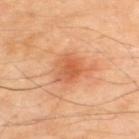Findings:
• follow-up — imaged on a skin check; not biopsied
• lighting — cross-polarized illumination
• anatomic site — the upper back
• image source — total-body-photography crop, ~15 mm field of view
• subject — male, approximately 65 years of age